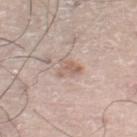Imaged during a routine full-body skin examination; the lesion was not biopsied and no histopathology is available.
The subject is a male aged around 70.
A 15 mm crop from a total-body photograph taken for skin-cancer surveillance.
The lesion is located on the left leg.
The tile uses white-light illumination.